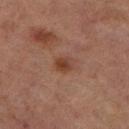Part of a total-body skin-imaging series; this lesion was reviewed on a skin check and was not flagged for biopsy. The total-body-photography lesion software estimated a footprint of about 4 mm², an eccentricity of roughly 0.6, and a shape-asymmetry score of about 0.15 (0 = symmetric). The software also gave a lesion color around L≈35 a*≈20 b*≈26 in CIELAB, a lesion–skin lightness drop of about 8, and a lesion-to-skin contrast of about 7.5 (normalized; higher = more distinct). And it measured a border-irregularity index near 1.5/10 and radial color variation of about 1.5. The software also gave a classifier nevus-likeness of about 90/100. Cropped from a whole-body photographic skin survey; the tile spans about 15 mm. Measured at roughly 2.5 mm in maximum diameter. From the leg. A female patient, aged 58–62.